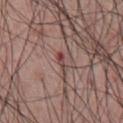Notes:
- biopsy status — total-body-photography surveillance lesion; no biopsy
- patient — male, aged approximately 55
- lighting — white-light
- lesion size — about 2.5 mm
- image — ~15 mm crop, total-body skin-cancer survey
- body site — the chest